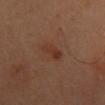Impression: This lesion was catalogued during total-body skin photography and was not selected for biopsy. Background: A male patient aged approximately 65. The lesion is located on the mid back. A 15 mm close-up tile from a total-body photography series done for melanoma screening. The lesion-visualizer software estimated a mean CIELAB color near L≈34 a*≈21 b*≈28 and a normalized border contrast of about 6. And it measured a classifier nevus-likeness of about 20/100 and a detector confidence of about 100 out of 100 that the crop contains a lesion.